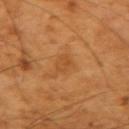notes: catalogued during a skin exam; not biopsied
automated metrics: a footprint of about 4.5 mm² and a shape-asymmetry score of about 0.25 (0 = symmetric); roughly 6 lightness units darker than nearby skin; border irregularity of about 2.5 on a 0–10 scale, a color-variation rating of about 3/10, and peripheral color asymmetry of about 1
tile lighting: cross-polarized
location: the left forearm
diameter: ≈3 mm
patient: male, aged 58–62
image source: 15 mm crop, total-body photography